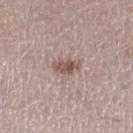The lesion was photographed on a routine skin check and not biopsied; there is no pathology result. Longest diameter approximately 3 mm. Located on the right lower leg. Automated image analysis of the tile measured a lesion area of about 4.5 mm², a shape eccentricity near 0.8, and a symmetry-axis asymmetry near 0.2. It also reported an average lesion color of about L≈53 a*≈18 b*≈22 (CIELAB), about 11 CIELAB-L* units darker than the surrounding skin, and a normalized lesion–skin contrast near 8. The software also gave border irregularity of about 2.5 on a 0–10 scale, a within-lesion color-variation index near 4.5/10, and peripheral color asymmetry of about 2. The analysis additionally found an automated nevus-likeness rating near 70 out of 100 and a lesion-detection confidence of about 100/100. A female subject about 65 years old. Imaged with white-light lighting. Cropped from a total-body skin-imaging series; the visible field is about 15 mm.